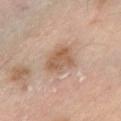size: ~4 mm (longest diameter)
image source: ~15 mm tile from a whole-body skin photo
TBP lesion metrics: a lesion area of about 9.5 mm² and a shape-asymmetry score of about 0.25 (0 = symmetric); an average lesion color of about L≈59 a*≈18 b*≈31 (CIELAB), about 10 CIELAB-L* units darker than the surrounding skin, and a normalized lesion–skin contrast near 7
site: the right forearm
subject: male, aged 53 to 57
tile lighting: cross-polarized